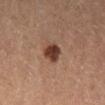Captured during whole-body skin photography for melanoma surveillance; the lesion was not biopsied. From the left lower leg. The total-body-photography lesion software estimated a normalized border contrast of about 11.5. The software also gave internal color variation of about 2.5 on a 0–10 scale and peripheral color asymmetry of about 1. The patient is a female aged approximately 60. The tile uses cross-polarized illumination. A 15 mm close-up tile from a total-body photography series done for melanoma screening.